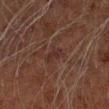{"biopsy_status": "not biopsied; imaged during a skin examination", "image": {"source": "total-body photography crop", "field_of_view_mm": 15}, "automated_metrics": {"cielab_L": 21, "cielab_a": 15, "cielab_b": 16, "vs_skin_darker_L": 4.0, "border_irregularity_0_10": 4.0, "color_variation_0_10": 1.5, "peripheral_color_asymmetry": 0.5}, "site": "left forearm", "patient": {"sex": "male", "age_approx": 60}, "lighting": "cross-polarized", "lesion_size": {"long_diameter_mm_approx": 3.0}}